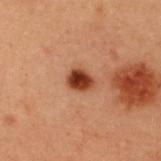notes=no biopsy performed (imaged during a skin exam); image source=~15 mm crop, total-body skin-cancer survey; subject=female, roughly 40 years of age; body site=the upper back.